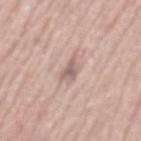workup — imaged on a skin check; not biopsied
patient — male, in their 70s
lesion diameter — about 3 mm
acquisition — 15 mm crop, total-body photography
illumination — white-light
site — the mid back